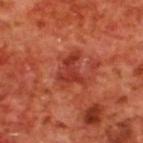biopsy_status: not biopsied; imaged during a skin examination
site: upper back
patient:
  sex: male
  age_approx: 70
image:
  source: total-body photography crop
  field_of_view_mm: 15
lesion_size:
  long_diameter_mm_approx: 4.0